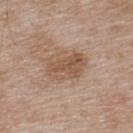Assessment: This lesion was catalogued during total-body skin photography and was not selected for biopsy. Background: The lesion-visualizer software estimated a lesion area of about 12 mm², a shape eccentricity near 0.8, and a shape-asymmetry score of about 0.25 (0 = symmetric). The analysis additionally found about 9 CIELAB-L* units darker than the surrounding skin and a normalized lesion–skin contrast near 7. The analysis additionally found a border-irregularity index near 3/10, internal color variation of about 4.5 on a 0–10 scale, and radial color variation of about 2. Imaged with white-light lighting. Approximately 5 mm at its widest. A male patient approximately 55 years of age. A roughly 15 mm field-of-view crop from a total-body skin photograph. On the back.